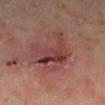biopsy_status: not biopsied; imaged during a skin examination
lesion_size:
  long_diameter_mm_approx: 6.0
image:
  source: total-body photography crop
  field_of_view_mm: 15
automated_metrics:
  cielab_L: 37
  cielab_a: 23
  cielab_b: 21
  vs_skin_contrast_norm: 7.0
  border_irregularity_0_10: 7.0
  color_variation_0_10: 7.0
  peripheral_color_asymmetry: 2.5
  nevus_likeness_0_100: 0
patient:
  sex: male
  age_approx: 60
site: left lower leg
lighting: cross-polarized